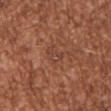The lesion was photographed on a routine skin check and not biopsied; there is no pathology result. A male patient in their mid- to late 40s. Cropped from a total-body skin-imaging series; the visible field is about 15 mm. From the right upper arm.